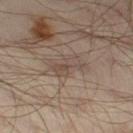No biopsy was performed on this lesion — it was imaged during a full skin examination and was not determined to be concerning.
Cropped from a whole-body photographic skin survey; the tile spans about 15 mm.
A male subject in their mid-60s.
On the left thigh.
The lesion's longest dimension is about 3.5 mm.